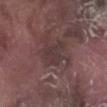* follow-up · imaged on a skin check; not biopsied
* diameter · ~3.5 mm (longest diameter)
* lighting · white-light
* subject · male, aged 73 to 77
* location · the right lower leg
* image · ~15 mm tile from a whole-body skin photo
* TBP lesion metrics · a border-irregularity rating of about 3.5/10 and a color-variation rating of about 2.5/10